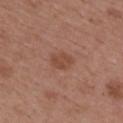biopsy status — no biopsy performed (imaged during a skin exam)
image — ~15 mm tile from a whole-body skin photo
body site — the upper back
subject — female, aged 48–52